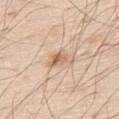workup = imaged on a skin check; not biopsied | image = ~15 mm tile from a whole-body skin photo | subject = male, approximately 80 years of age | tile lighting = white-light illumination | anatomic site = the upper back.